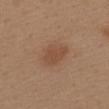The lesion was photographed on a routine skin check and not biopsied; there is no pathology result.
Measured at roughly 4 mm in maximum diameter.
A female patient aged 38 to 42.
Captured under white-light illumination.
A close-up tile cropped from a whole-body skin photograph, about 15 mm across.
From the upper back.